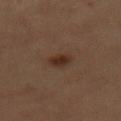{
  "biopsy_status": "not biopsied; imaged during a skin examination",
  "patient": {
    "sex": "female",
    "age_approx": 60
  },
  "image": {
    "source": "total-body photography crop",
    "field_of_view_mm": 15
  },
  "lighting": "cross-polarized",
  "site": "front of the torso",
  "automated_metrics": {
    "area_mm2_approx": 5.0,
    "eccentricity": 0.3,
    "nevus_likeness_0_100": 95,
    "lesion_detection_confidence_0_100": 100
  },
  "lesion_size": {
    "long_diameter_mm_approx": 2.5
  }
}